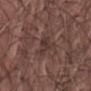Clinical impression:
The lesion was tiled from a total-body skin photograph and was not biopsied.
Image and clinical context:
A male subject roughly 55 years of age. Cropped from a total-body skin-imaging series; the visible field is about 15 mm. The lesion-visualizer software estimated a mean CIELAB color near L≈34 a*≈16 b*≈18, about 7 CIELAB-L* units darker than the surrounding skin, and a normalized border contrast of about 7. It also reported border irregularity of about 4.5 on a 0–10 scale, a color-variation rating of about 2/10, and radial color variation of about 1. The software also gave lesion-presence confidence of about 50/100. Imaged with white-light lighting. The lesion is on the abdomen. The lesion's longest dimension is about 3 mm.